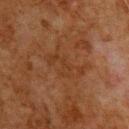biopsy status: total-body-photography surveillance lesion; no biopsy | site: the upper back | imaging modality: ~15 mm tile from a whole-body skin photo | subject: male, about 80 years old | automated metrics: a lesion area of about 10 mm²; a mean CIELAB color near L≈29 a*≈18 b*≈27, roughly 4 lightness units darker than nearby skin, and a normalized lesion–skin contrast near 4.5; border irregularity of about 6.5 on a 0–10 scale, a within-lesion color-variation index near 2.5/10, and radial color variation of about 1; a classifier nevus-likeness of about 0/100 and a lesion-detection confidence of about 100/100.